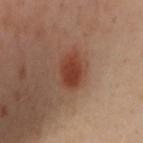Clinical impression: Captured during whole-body skin photography for melanoma surveillance; the lesion was not biopsied. Context: The recorded lesion diameter is about 4 mm. Imaged with cross-polarized lighting. The patient is a female in their 40s. The lesion-visualizer software estimated a classifier nevus-likeness of about 100/100 and a lesion-detection confidence of about 100/100. Cropped from a whole-body photographic skin survey; the tile spans about 15 mm. Located on the left arm.